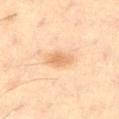notes = total-body-photography surveillance lesion; no biopsy | image = ~15 mm tile from a whole-body skin photo | diameter = ≈3.5 mm | patient = male, aged around 60 | illumination = cross-polarized illumination | site = the right thigh.